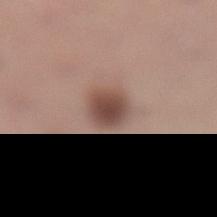- automated lesion analysis: a mean CIELAB color near L≈47 a*≈19 b*≈24, a lesion–skin lightness drop of about 13, and a normalized lesion–skin contrast near 9.5
- illumination: white-light illumination
- location: the left lower leg
- patient: female, roughly 30 years of age
- lesion size: ~3 mm (longest diameter)
- image source: total-body-photography crop, ~15 mm field of view